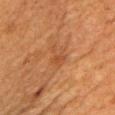Impression:
Part of a total-body skin-imaging series; this lesion was reviewed on a skin check and was not flagged for biopsy.
Context:
A female patient, in their mid-50s. The lesion is on the right upper arm. Automated image analysis of the tile measured a footprint of about 4 mm², an eccentricity of roughly 0.8, and two-axis asymmetry of about 0.5. A 15 mm close-up tile from a total-body photography series done for melanoma screening. The recorded lesion diameter is about 3.5 mm. Imaged with cross-polarized lighting.